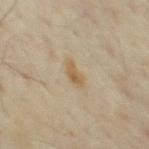No biopsy was performed on this lesion — it was imaged during a full skin examination and was not determined to be concerning. The subject is a male aged 63 to 67. A 15 mm close-up tile from a total-body photography series done for melanoma screening. Imaged with cross-polarized lighting. The lesion is located on the chest.